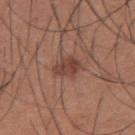No biopsy was performed on this lesion — it was imaged during a full skin examination and was not determined to be concerning.